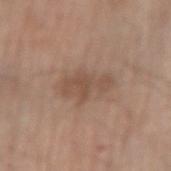This image is a 15 mm lesion crop taken from a total-body photograph.
The tile uses white-light illumination.
Approximately 4.5 mm at its widest.
Located on the right forearm.
A male patient about 70 years old.